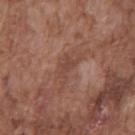Assessment:
Recorded during total-body skin imaging; not selected for excision or biopsy.
Context:
About 5 mm across. A region of skin cropped from a whole-body photographic capture, roughly 15 mm wide. Located on the chest. The lesion-visualizer software estimated an area of roughly 7 mm², an eccentricity of roughly 0.9, and a symmetry-axis asymmetry near 0.5. The analysis additionally found a mean CIELAB color near L≈45 a*≈21 b*≈26, about 6 CIELAB-L* units darker than the surrounding skin, and a normalized border contrast of about 5. And it measured border irregularity of about 7 on a 0–10 scale, internal color variation of about 1.5 on a 0–10 scale, and radial color variation of about 0.5. Captured under white-light illumination. A male subject in their mid-70s.